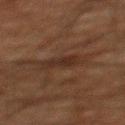| key | value |
|---|---|
| biopsy status | catalogued during a skin exam; not biopsied |
| illumination | cross-polarized |
| imaging modality | ~15 mm crop, total-body skin-cancer survey |
| diameter | ≈3.5 mm |
| subject | male, aged approximately 60 |
| body site | the mid back |